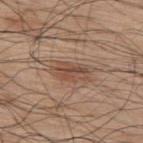lighting: white-light
site: back
patient:
  sex: male
  age_approx: 70
image:
  source: total-body photography crop
  field_of_view_mm: 15
lesion_size:
  long_diameter_mm_approx: 4.0
automated_metrics:
  eccentricity: 0.85
  shape_asymmetry: 0.3
  border_irregularity_0_10: 4.0
  color_variation_0_10: 5.0
  lesion_detection_confidence_0_100: 100